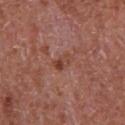Captured during whole-body skin photography for melanoma surveillance; the lesion was not biopsied.
A close-up tile cropped from a whole-body skin photograph, about 15 mm across.
Located on the chest.
Captured under white-light illumination.
A male patient, in their mid- to late 60s.